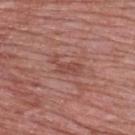Clinical summary: Cropped from a whole-body photographic skin survey; the tile spans about 15 mm. A male patient, roughly 50 years of age. The lesion is on the upper back.